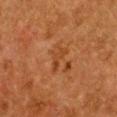Captured during whole-body skin photography for melanoma surveillance; the lesion was not biopsied. Automated image analysis of the tile measured an average lesion color of about L≈36 a*≈22 b*≈34 (CIELAB) and a lesion–skin lightness drop of about 6. The analysis additionally found border irregularity of about 6 on a 0–10 scale, a within-lesion color-variation index near 0/10, and radial color variation of about 0. The software also gave a detector confidence of about 100 out of 100 that the crop contains a lesion. A female subject aged 48–52. Located on the chest. A roughly 15 mm field-of-view crop from a total-body skin photograph. This is a cross-polarized tile.